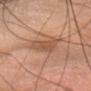{"biopsy_status": "not biopsied; imaged during a skin examination", "patient": {"sex": "female", "age_approx": 55}, "lesion_size": {"long_diameter_mm_approx": 4.5}, "image": {"source": "total-body photography crop", "field_of_view_mm": 15}, "lighting": "white-light", "automated_metrics": {"area_mm2_approx": 9.5, "eccentricity": 0.8, "shape_asymmetry": 0.35}, "site": "head or neck"}